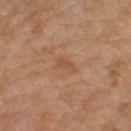Assessment:
Imaged during a routine full-body skin examination; the lesion was not biopsied and no histopathology is available.
Image and clinical context:
The lesion is on the left lower leg. A female patient aged 58–62. The lesion's longest dimension is about 2.5 mm. Captured under cross-polarized illumination. A 15 mm crop from a total-body photograph taken for skin-cancer surveillance.